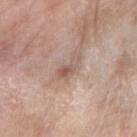This lesion was catalogued during total-body skin photography and was not selected for biopsy.
A roughly 15 mm field-of-view crop from a total-body skin photograph.
The subject is a female aged around 70.
The lesion is on the right forearm.